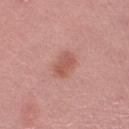Recorded during total-body skin imaging; not selected for excision or biopsy.
The recorded lesion diameter is about 3.5 mm.
The patient is a female in their mid- to late 50s.
The lesion is located on the leg.
A 15 mm close-up extracted from a 3D total-body photography capture.
Captured under white-light illumination.